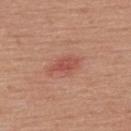Q: Was this lesion biopsied?
A: imaged on a skin check; not biopsied